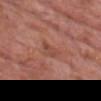Clinical impression:
Recorded during total-body skin imaging; not selected for excision or biopsy.
Acquisition and patient details:
Cropped from a whole-body photographic skin survey; the tile spans about 15 mm. Automated tile analysis of the lesion measured a lesion area of about 5 mm², an outline eccentricity of about 0.75 (0 = round, 1 = elongated), and a symmetry-axis asymmetry near 0.35. The analysis additionally found border irregularity of about 3.5 on a 0–10 scale, a color-variation rating of about 3.5/10, and peripheral color asymmetry of about 1. Located on the chest. A male patient aged approximately 60. Imaged with white-light lighting. The recorded lesion diameter is about 3 mm.